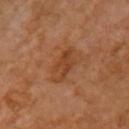No biopsy was performed on this lesion — it was imaged during a full skin examination and was not determined to be concerning. The subject is a female in their mid- to late 60s. From the chest. The lesion's longest dimension is about 4.5 mm. Automated image analysis of the tile measured a lesion–skin lightness drop of about 8 and a lesion-to-skin contrast of about 6.5 (normalized; higher = more distinct). The software also gave a border-irregularity index near 5/10 and peripheral color asymmetry of about 0.5. The analysis additionally found an automated nevus-likeness rating near 10 out of 100 and a detector confidence of about 100 out of 100 that the crop contains a lesion. A lesion tile, about 15 mm wide, cut from a 3D total-body photograph.